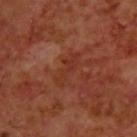A region of skin cropped from a whole-body photographic capture, roughly 15 mm wide. Captured under cross-polarized illumination. Located on the upper back. Approximately 4.5 mm at its widest. A male subject about 70 years old.